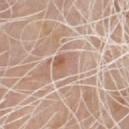Recorded during total-body skin imaging; not selected for excision or biopsy.